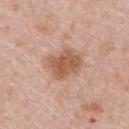Assessment:
Recorded during total-body skin imaging; not selected for excision or biopsy.
Acquisition and patient details:
The patient is a female roughly 45 years of age. Located on the upper back. A 15 mm crop from a total-body photograph taken for skin-cancer surveillance. Imaged with white-light lighting. The lesion's longest dimension is about 4 mm.